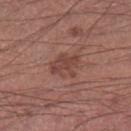This is a white-light tile.
Located on the right lower leg.
An algorithmic analysis of the crop reported a footprint of about 6.5 mm², an outline eccentricity of about 0.65 (0 = round, 1 = elongated), and a shape-asymmetry score of about 0.3 (0 = symmetric). The analysis additionally found border irregularity of about 4.5 on a 0–10 scale, internal color variation of about 2.5 on a 0–10 scale, and radial color variation of about 1. It also reported a classifier nevus-likeness of about 20/100 and a detector confidence of about 100 out of 100 that the crop contains a lesion.
The subject is a male aged 43–47.
Measured at roughly 3 mm in maximum diameter.
A roughly 15 mm field-of-view crop from a total-body skin photograph.